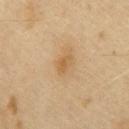The lesion was tiled from a total-body skin photograph and was not biopsied.
The patient is a male aged 63 to 67.
The recorded lesion diameter is about 3 mm.
A close-up tile cropped from a whole-body skin photograph, about 15 mm across.
Imaged with cross-polarized lighting.